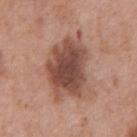Assessment:
Imaged during a routine full-body skin examination; the lesion was not biopsied and no histopathology is available.
Clinical summary:
Captured under white-light illumination. A male subject, approximately 70 years of age. A close-up tile cropped from a whole-body skin photograph, about 15 mm across. From the chest.